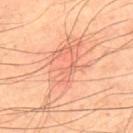Clinical impression: Imaged during a routine full-body skin examination; the lesion was not biopsied and no histopathology is available. Context: This is a cross-polarized tile. A close-up tile cropped from a whole-body skin photograph, about 15 mm across. The lesion's longest dimension is about 10.5 mm. A male patient approximately 50 years of age. The lesion is on the upper back.